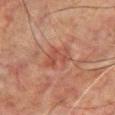| field | value |
|---|---|
| notes | total-body-photography surveillance lesion; no biopsy |
| lighting | cross-polarized illumination |
| automated metrics | an area of roughly 7 mm², an eccentricity of roughly 0.8, and a symmetry-axis asymmetry near 0.35; a normalized lesion–skin contrast near 5.5; lesion-presence confidence of about 100/100 |
| size | about 4 mm |
| subject | male, about 65 years old |
| image | ~15 mm crop, total-body skin-cancer survey |
| site | the chest |